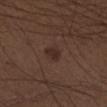<case>
<biopsy_status>not biopsied; imaged during a skin examination</biopsy_status>
<image>
  <source>total-body photography crop</source>
  <field_of_view_mm>15</field_of_view_mm>
</image>
<site>right lower leg</site>
<lighting>white-light</lighting>
<patient>
  <sex>male</sex>
  <age_approx>50</age_approx>
</patient>
<lesion_size>
  <long_diameter_mm_approx>2.5</long_diameter_mm_approx>
</lesion_size>
<automated_metrics>
  <cielab_L>28</cielab_L>
  <cielab_a>16</cielab_a>
  <cielab_b>20</cielab_b>
  <vs_skin_darker_L>6.0</vs_skin_darker_L>
  <vs_skin_contrast_norm>7.0</vs_skin_contrast_norm>
  <nevus_likeness_0_100>75</nevus_likeness_0_100>
  <lesion_detection_confidence_0_100>100</lesion_detection_confidence_0_100>
</automated_metrics>
</case>